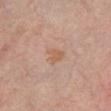<case>
<biopsy_status>not biopsied; imaged during a skin examination</biopsy_status>
<lesion_size>
  <long_diameter_mm_approx>2.5</long_diameter_mm_approx>
</lesion_size>
<automated_metrics>
  <area_mm2_approx>4.0</area_mm2_approx>
  <eccentricity>0.65</eccentricity>
  <shape_asymmetry>0.35</shape_asymmetry>
  <border_irregularity_0_10>3.5</border_irregularity_0_10>
  <color_variation_0_10>2.0</color_variation_0_10>
  <peripheral_color_asymmetry>1.0</peripheral_color_asymmetry>
</automated_metrics>
<image>
  <source>total-body photography crop</source>
  <field_of_view_mm>15</field_of_view_mm>
</image>
<patient>
  <sex>male</sex>
  <age_approx>60</age_approx>
</patient>
<site>right lower leg</site>
<lighting>cross-polarized</lighting>
</case>